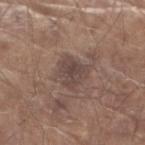Q: Was this lesion biopsied?
A: imaged on a skin check; not biopsied
Q: Illumination type?
A: white-light
Q: What are the patient's age and sex?
A: male, aged around 80
Q: Where on the body is the lesion?
A: the right upper arm
Q: Lesion size?
A: ≈3 mm
Q: What kind of image is this?
A: ~15 mm crop, total-body skin-cancer survey